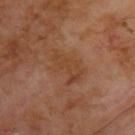Recorded during total-body skin imaging; not selected for excision or biopsy.
Cropped from a whole-body photographic skin survey; the tile spans about 15 mm.
Measured at roughly 4.5 mm in maximum diameter.
From the back.
A male subject, approximately 70 years of age.
The total-body-photography lesion software estimated an eccentricity of roughly 0.8 and a shape-asymmetry score of about 0.4 (0 = symmetric). The analysis additionally found about 5 CIELAB-L* units darker than the surrounding skin and a normalized border contrast of about 5.5. The analysis additionally found a color-variation rating of about 4/10 and a peripheral color-asymmetry measure near 1.5. The software also gave a nevus-likeness score of about 0/100 and a lesion-detection confidence of about 100/100.